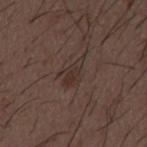No biopsy was performed on this lesion — it was imaged during a full skin examination and was not determined to be concerning.
The subject is a male in their 50s.
A 15 mm close-up tile from a total-body photography series done for melanoma screening.
Imaged with white-light lighting.
Approximately 3.5 mm at its widest.
Automated image analysis of the tile measured a border-irregularity index near 3.5/10, internal color variation of about 2 on a 0–10 scale, and radial color variation of about 0.5.
The lesion is on the mid back.